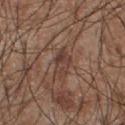Part of a total-body skin-imaging series; this lesion was reviewed on a skin check and was not flagged for biopsy.
About 3.5 mm across.
The lesion is on the front of the torso.
The tile uses white-light illumination.
The subject is a male about 55 years old.
A 15 mm crop from a total-body photograph taken for skin-cancer surveillance.